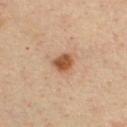Q: Was this lesion biopsied?
A: imaged on a skin check; not biopsied
Q: How was the tile lit?
A: cross-polarized illumination
Q: What kind of image is this?
A: total-body-photography crop, ~15 mm field of view
Q: Where on the body is the lesion?
A: the chest
Q: Who is the patient?
A: male, aged around 35
Q: How large is the lesion?
A: ≈2.5 mm
Q: Automated lesion metrics?
A: a lesion area of about 5 mm², a shape eccentricity near 0.45, and a symmetry-axis asymmetry near 0.25; internal color variation of about 2.5 on a 0–10 scale